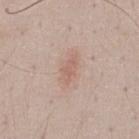This lesion was catalogued during total-body skin photography and was not selected for biopsy. A 15 mm crop from a total-body photograph taken for skin-cancer surveillance. Longest diameter approximately 3.5 mm. Captured under white-light illumination. The lesion is located on the front of the torso. A male subject, roughly 50 years of age. An algorithmic analysis of the crop reported a footprint of about 4 mm² and a shape-asymmetry score of about 0.35 (0 = symmetric). It also reported a border-irregularity rating of about 3.5/10 and radial color variation of about 0.5.